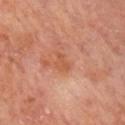<tbp_lesion>
<biopsy_status>not biopsied; imaged during a skin examination</biopsy_status>
<lighting>cross-polarized</lighting>
<lesion_size>
  <long_diameter_mm_approx>2.5</long_diameter_mm_approx>
</lesion_size>
<image>
  <source>total-body photography crop</source>
  <field_of_view_mm>15</field_of_view_mm>
</image>
<automated_metrics>
  <cielab_L>52</cielab_L>
  <cielab_a>25</cielab_a>
  <cielab_b>34</cielab_b>
  <vs_skin_darker_L>6.0</vs_skin_darker_L>
  <vs_skin_contrast_norm>5.5</vs_skin_contrast_norm>
</automated_metrics>
<patient>
  <sex>male</sex>
  <age_approx>65</age_approx>
</patient>
<site>arm</site>
</tbp_lesion>